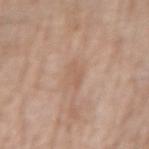| field | value |
|---|---|
| follow-up | imaged on a skin check; not biopsied |
| patient | male, in their 70s |
| body site | the right forearm |
| image | total-body-photography crop, ~15 mm field of view |